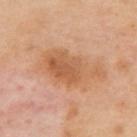Q: What is the lesion's diameter?
A: about 7 mm
Q: How was this image acquired?
A: ~15 mm tile from a whole-body skin photo
Q: Patient demographics?
A: female, aged 38–42
Q: Where on the body is the lesion?
A: the upper back
Q: What lighting was used for the tile?
A: cross-polarized illumination
Q: Automated lesion metrics?
A: border irregularity of about 4.5 on a 0–10 scale, a within-lesion color-variation index near 5/10, and peripheral color asymmetry of about 1.5; an automated nevus-likeness rating near 25 out of 100 and a detector confidence of about 100 out of 100 that the crop contains a lesion